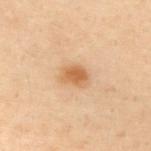Clinical impression: The lesion was photographed on a routine skin check and not biopsied; there is no pathology result.